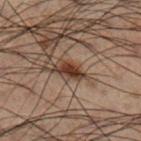workup: no biopsy performed (imaged during a skin exam) | site: the right lower leg | patient: male, roughly 50 years of age | imaging modality: total-body-photography crop, ~15 mm field of view.